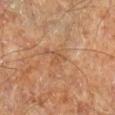Assessment: Recorded during total-body skin imaging; not selected for excision or biopsy. Acquisition and patient details: The tile uses cross-polarized illumination. On the right lower leg. A roughly 15 mm field-of-view crop from a total-body skin photograph. The subject is a male aged around 60. The lesion's longest dimension is about 2 mm.